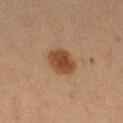Imaged during a routine full-body skin examination; the lesion was not biopsied and no histopathology is available.
The recorded lesion diameter is about 3.5 mm.
This is a cross-polarized tile.
The lesion is on the right thigh.
An algorithmic analysis of the crop reported an outline eccentricity of about 0.5 (0 = round, 1 = elongated) and two-axis asymmetry of about 0.15. It also reported a nevus-likeness score of about 100/100.
The subject is a female in their 20s.
A 15 mm close-up tile from a total-body photography series done for melanoma screening.